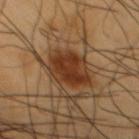Part of a total-body skin-imaging series; this lesion was reviewed on a skin check and was not flagged for biopsy. From the chest. The lesion's longest dimension is about 5 mm. A male subject about 60 years old. Cropped from a total-body skin-imaging series; the visible field is about 15 mm.